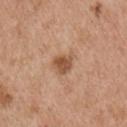Q: Where on the body is the lesion?
A: the right upper arm
Q: What is the lesion's diameter?
A: about 3 mm
Q: What is the imaging modality?
A: 15 mm crop, total-body photography
Q: Patient demographics?
A: male, approximately 55 years of age